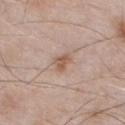Q: Is there a histopathology result?
A: no biopsy performed (imaged during a skin exam)
Q: Where on the body is the lesion?
A: the front of the torso
Q: What lighting was used for the tile?
A: white-light
Q: What did automated image analysis measure?
A: a within-lesion color-variation index near 2/10 and peripheral color asymmetry of about 0.5; a classifier nevus-likeness of about 40/100 and lesion-presence confidence of about 100/100
Q: How was this image acquired?
A: ~15 mm crop, total-body skin-cancer survey
Q: Patient demographics?
A: male, aged around 55
Q: Lesion size?
A: ~2.5 mm (longest diameter)